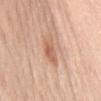Captured during whole-body skin photography for melanoma surveillance; the lesion was not biopsied. A female subject, approximately 70 years of age. Longest diameter approximately 4 mm. The lesion-visualizer software estimated a lesion area of about 7 mm², an eccentricity of roughly 0.9, and a shape-asymmetry score of about 0.35 (0 = symmetric). The software also gave a lesion color around L≈63 a*≈22 b*≈32 in CIELAB and a lesion-to-skin contrast of about 6.5 (normalized; higher = more distinct). From the mid back. Cropped from a total-body skin-imaging series; the visible field is about 15 mm. This is a white-light tile.